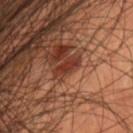<record>
  <biopsy_status>not biopsied; imaged during a skin examination</biopsy_status>
  <patient>
    <sex>male</sex>
    <age_approx>40</age_approx>
  </patient>
  <lesion_size>
    <long_diameter_mm_approx>3.5</long_diameter_mm_approx>
  </lesion_size>
  <automated_metrics>
    <area_mm2_approx>4.0</area_mm2_approx>
    <border_irregularity_0_10>3.5</border_irregularity_0_10>
    <peripheral_color_asymmetry>1.0</peripheral_color_asymmetry>
    <nevus_likeness_0_100>0</nevus_likeness_0_100>
    <lesion_detection_confidence_0_100>85</lesion_detection_confidence_0_100>
  </automated_metrics>
  <lighting>cross-polarized</lighting>
  <site>head or neck</site>
  <image>
    <source>total-body photography crop</source>
    <field_of_view_mm>15</field_of_view_mm>
  </image>
</record>